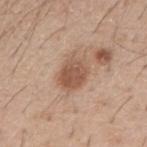Captured during whole-body skin photography for melanoma surveillance; the lesion was not biopsied.
Captured under white-light illumination.
A lesion tile, about 15 mm wide, cut from a 3D total-body photograph.
The lesion is on the arm.
About 4 mm across.
Automated tile analysis of the lesion measured an eccentricity of roughly 0.6 and a shape-asymmetry score of about 0.2 (0 = symmetric). It also reported a lesion color around L≈55 a*≈19 b*≈30 in CIELAB, about 11 CIELAB-L* units darker than the surrounding skin, and a normalized border contrast of about 7.5.
The patient is a male roughly 40 years of age.